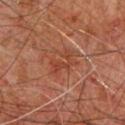<case>
  <biopsy_status>not biopsied; imaged during a skin examination</biopsy_status>
  <patient>
    <sex>male</sex>
    <age_approx>60</age_approx>
  </patient>
  <automated_metrics>
    <vs_skin_darker_L>6.0</vs_skin_darker_L>
    <lesion_detection_confidence_0_100>95</lesion_detection_confidence_0_100>
  </automated_metrics>
  <site>upper back</site>
  <lighting>cross-polarized</lighting>
  <image>
    <source>total-body photography crop</source>
    <field_of_view_mm>15</field_of_view_mm>
  </image>
  <lesion_size>
    <long_diameter_mm_approx>3.5</long_diameter_mm_approx>
  </lesion_size>
</case>